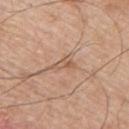Imaged during a routine full-body skin examination; the lesion was not biopsied and no histopathology is available.
Captured under white-light illumination.
Automated image analysis of the tile measured border irregularity of about 4.5 on a 0–10 scale and radial color variation of about 0. The software also gave a classifier nevus-likeness of about 0/100.
The subject is a male about 55 years old.
The lesion is located on the upper back.
Approximately 3 mm at its widest.
Cropped from a total-body skin-imaging series; the visible field is about 15 mm.